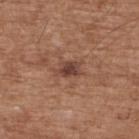biopsy_status: not biopsied; imaged during a skin examination
site: upper back
patient:
  sex: male
  age_approx: 75
lighting: white-light
image:
  source: total-body photography crop
  field_of_view_mm: 15
lesion_size:
  long_diameter_mm_approx: 3.0
automated_metrics:
  area_mm2_approx: 4.5
  eccentricity: 0.8
  shape_asymmetry: 0.25
  cielab_L: 42
  cielab_a: 21
  cielab_b: 26
  vs_skin_darker_L: 10.0
  vs_skin_contrast_norm: 8.5
  nevus_likeness_0_100: 60